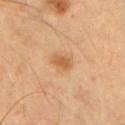{"biopsy_status": "not biopsied; imaged during a skin examination", "image": {"source": "total-body photography crop", "field_of_view_mm": 15}, "patient": {"sex": "male", "age_approx": 55}, "site": "chest", "lighting": "cross-polarized"}